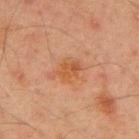| field | value |
|---|---|
| biopsy status | imaged on a skin check; not biopsied |
| TBP lesion metrics | lesion-presence confidence of about 100/100 |
| illumination | cross-polarized |
| patient | male, about 45 years old |
| size | about 2.5 mm |
| image source | total-body-photography crop, ~15 mm field of view |
| body site | the upper back |